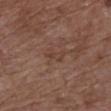image: ~15 mm crop, total-body skin-cancer survey
tile lighting: white-light illumination
subject: female, in their 80s
body site: the chest
image-analysis metrics: roughly 5 lightness units darker than nearby skin and a lesion-to-skin contrast of about 5 (normalized; higher = more distinct)
lesion size: about 2.5 mm